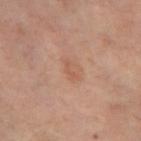{
  "biopsy_status": "not biopsied; imaged during a skin examination",
  "patient": {
    "sex": "female",
    "age_approx": 55
  },
  "image": {
    "source": "total-body photography crop",
    "field_of_view_mm": 15
  },
  "lighting": "cross-polarized",
  "site": "left thigh",
  "lesion_size": {
    "long_diameter_mm_approx": 2.5
  }
}